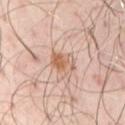{"biopsy_status": "not biopsied; imaged during a skin examination", "lighting": "cross-polarized", "automated_metrics": {"vs_skin_darker_L": 10.0, "vs_skin_contrast_norm": 7.0, "border_irregularity_0_10": 2.5, "color_variation_0_10": 6.5, "peripheral_color_asymmetry": 2.0, "nevus_likeness_0_100": 80, "lesion_detection_confidence_0_100": 100}, "image": {"source": "total-body photography crop", "field_of_view_mm": 15}, "patient": {"sex": "male", "age_approx": 50}, "site": "abdomen"}